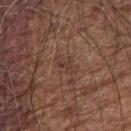biopsy status: no biopsy performed (imaged during a skin exam) | illumination: white-light illumination | body site: the right forearm | patient: male, approximately 65 years of age | automated lesion analysis: an eccentricity of roughly 0.85 and two-axis asymmetry of about 0.55; a detector confidence of about 85 out of 100 that the crop contains a lesion | image: ~15 mm crop, total-body skin-cancer survey | diameter: ≈2.5 mm.